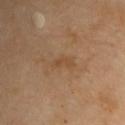A roughly 15 mm field-of-view crop from a total-body skin photograph. A male patient, in their mid- to late 50s. On the chest. This is a cross-polarized tile. Approximately 3 mm at its widest.